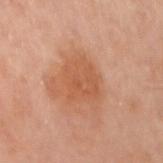- notes — catalogued during a skin exam; not biopsied
- body site — the left arm
- image source — 15 mm crop, total-body photography
- subject — female, roughly 60 years of age